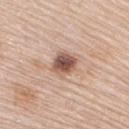biopsy status: catalogued during a skin exam; not biopsied | site: the right upper arm | tile lighting: white-light | patient: female, aged 63 to 67 | imaging modality: total-body-photography crop, ~15 mm field of view.